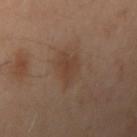{"biopsy_status": "not biopsied; imaged during a skin examination"}